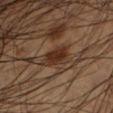Recorded during total-body skin imaging; not selected for excision or biopsy. Approximately 3.5 mm at its widest. An algorithmic analysis of the crop reported a footprint of about 6 mm², a shape eccentricity near 0.75, and a symmetry-axis asymmetry near 0.2. It also reported a border-irregularity rating of about 2/10, a color-variation rating of about 2.5/10, and peripheral color asymmetry of about 1. It also reported a classifier nevus-likeness of about 95/100 and a detector confidence of about 95 out of 100 that the crop contains a lesion. A 15 mm close-up extracted from a 3D total-body photography capture. A male patient aged 43 to 47. Imaged with cross-polarized lighting. From the left lower leg.